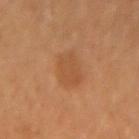This lesion was catalogued during total-body skin photography and was not selected for biopsy. The lesion's longest dimension is about 4 mm. Located on the left forearm. This is a cross-polarized tile. A region of skin cropped from a whole-body photographic capture, roughly 15 mm wide. Automated image analysis of the tile measured an area of roughly 8 mm² and two-axis asymmetry of about 0.2. A female subject, about 35 years old.